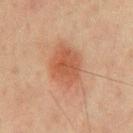notes=no biopsy performed (imaged during a skin exam)
automated lesion analysis=a footprint of about 18 mm², a shape eccentricity near 0.8, and two-axis asymmetry of about 0.15; an average lesion color of about L≈44 a*≈20 b*≈28 (CIELAB), a lesion–skin lightness drop of about 8, and a normalized lesion–skin contrast near 6.5; a nevus-likeness score of about 100/100 and a lesion-detection confidence of about 100/100
subject=male, about 65 years old
illumination=cross-polarized
imaging modality=total-body-photography crop, ~15 mm field of view
diameter=~6 mm (longest diameter)
anatomic site=the chest